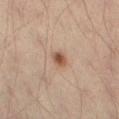Recorded during total-body skin imaging; not selected for excision or biopsy. Located on the left thigh. The patient is a male aged 28 to 32. Cropped from a whole-body photographic skin survey; the tile spans about 15 mm. This is a cross-polarized tile. The lesion's longest dimension is about 2 mm.